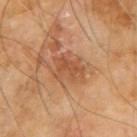{
  "biopsy_status": "not biopsied; imaged during a skin examination",
  "lesion_size": {
    "long_diameter_mm_approx": 3.5
  },
  "site": "arm",
  "patient": {
    "sex": "male",
    "age_approx": 65
  },
  "image": {
    "source": "total-body photography crop",
    "field_of_view_mm": 15
  }
}